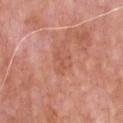notes=no biopsy performed (imaged during a skin exam) | patient=male, about 80 years old | illumination=white-light | imaging modality=~15 mm tile from a whole-body skin photo | automated lesion analysis=a border-irregularity rating of about 7/10, internal color variation of about 0 on a 0–10 scale, and a peripheral color-asymmetry measure near 0 | body site=the front of the torso.